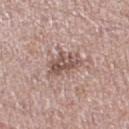No biopsy was performed on this lesion — it was imaged during a full skin examination and was not determined to be concerning.